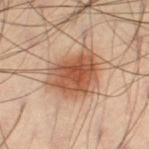workup=total-body-photography surveillance lesion; no biopsy | imaging modality=~15 mm tile from a whole-body skin photo | subject=male, about 40 years old | body site=the left thigh | automated lesion analysis=a lesion–skin lightness drop of about 14 and a normalized border contrast of about 9.5; a border-irregularity rating of about 2.5/10, internal color variation of about 5 on a 0–10 scale, and radial color variation of about 1.5 | lesion size=about 5.5 mm | lighting=cross-polarized illumination.